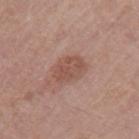- follow-up — catalogued during a skin exam; not biopsied
- diameter — about 4 mm
- location — the left upper arm
- image-analysis metrics — an average lesion color of about L≈51 a*≈21 b*≈26 (CIELAB); a lesion-detection confidence of about 100/100
- subject — female, aged approximately 50
- acquisition — 15 mm crop, total-body photography
- tile lighting — white-light